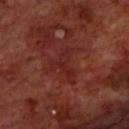{"biopsy_status": "not biopsied; imaged during a skin examination", "patient": {"sex": "male", "age_approx": 70}, "image": {"source": "total-body photography crop", "field_of_view_mm": 15}, "lesion_size": {"long_diameter_mm_approx": 4.5}, "automated_metrics": {"cielab_L": 25, "cielab_a": 27, "cielab_b": 24, "vs_skin_darker_L": 5.0, "vs_skin_contrast_norm": 5.5, "lesion_detection_confidence_0_100": 90}, "site": "back"}